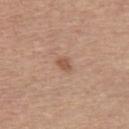The lesion was tiled from a total-body skin photograph and was not biopsied. From the upper back. A female patient, aged around 65. A 15 mm close-up tile from a total-body photography series done for melanoma screening. Imaged with white-light lighting.